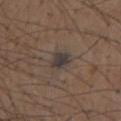Imaged during a routine full-body skin examination; the lesion was not biopsied and no histopathology is available.